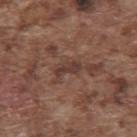workup: catalogued during a skin exam; not biopsied
tile lighting: white-light
location: the upper back
subject: male, about 75 years old
diameter: about 3.5 mm
imaging modality: total-body-photography crop, ~15 mm field of view
image-analysis metrics: an average lesion color of about L≈37 a*≈19 b*≈22 (CIELAB), a lesion–skin lightness drop of about 8, and a normalized lesion–skin contrast near 7.5; a border-irregularity rating of about 3.5/10 and internal color variation of about 1 on a 0–10 scale; a nevus-likeness score of about 0/100 and a lesion-detection confidence of about 60/100